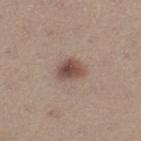Context: Automated image analysis of the tile measured a lesion color around L≈49 a*≈18 b*≈23 in CIELAB and a normalized border contrast of about 9. The software also gave a classifier nevus-likeness of about 95/100 and a detector confidence of about 100 out of 100 that the crop contains a lesion. A roughly 15 mm field-of-view crop from a total-body skin photograph. Captured under white-light illumination. A female subject, about 25 years old. The lesion is located on the left thigh.